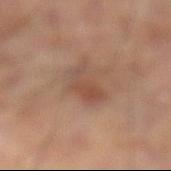Findings:
– follow-up · no biopsy performed (imaged during a skin exam)
– patient · male, aged around 55
– image · ~15 mm tile from a whole-body skin photo
– diameter · ≈4.5 mm
– anatomic site · the left lower leg
– TBP lesion metrics · an area of roughly 8 mm², an eccentricity of roughly 0.85, and two-axis asymmetry of about 0.4
– lighting · cross-polarized